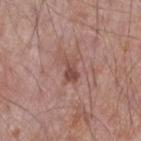The lesion is on the right forearm.
A roughly 15 mm field-of-view crop from a total-body skin photograph.
The subject is a male approximately 70 years of age.